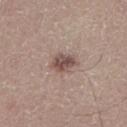Clinical impression:
This lesion was catalogued during total-body skin photography and was not selected for biopsy.
Acquisition and patient details:
A region of skin cropped from a whole-body photographic capture, roughly 15 mm wide. A male subject, roughly 45 years of age. Captured under white-light illumination. On the leg. The lesion-visualizer software estimated a nevus-likeness score of about 90/100.